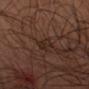Part of a total-body skin-imaging series; this lesion was reviewed on a skin check and was not flagged for biopsy.
Measured at roughly 3 mm in maximum diameter.
The total-body-photography lesion software estimated a within-lesion color-variation index near 0/10.
From the left upper arm.
A male patient, about 65 years old.
This is a cross-polarized tile.
A close-up tile cropped from a whole-body skin photograph, about 15 mm across.